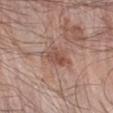The patient is a male roughly 70 years of age.
Cropped from a whole-body photographic skin survey; the tile spans about 15 mm.
The tile uses white-light illumination.
Located on the left forearm.